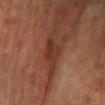Q: Was a biopsy performed?
A: total-body-photography surveillance lesion; no biopsy
Q: Lesion size?
A: ~4 mm (longest diameter)
Q: Automated lesion metrics?
A: a lesion color around L≈33 a*≈24 b*≈27 in CIELAB, about 8 CIELAB-L* units darker than the surrounding skin, and a normalized border contrast of about 7.5
Q: Where on the body is the lesion?
A: the right lower leg
Q: What lighting was used for the tile?
A: cross-polarized
Q: What is the imaging modality?
A: ~15 mm crop, total-body skin-cancer survey
Q: What are the patient's age and sex?
A: female, in their mid-60s